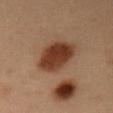<record>
<biopsy_status>not biopsied; imaged during a skin examination</biopsy_status>
<image>
  <source>total-body photography crop</source>
  <field_of_view_mm>15</field_of_view_mm>
</image>
<lighting>cross-polarized</lighting>
<automated_metrics>
  <eccentricity>0.7</eccentricity>
  <shape_asymmetry>0.15</shape_asymmetry>
</automated_metrics>
<site>mid back</site>
<lesion_size>
  <long_diameter_mm_approx>5.0</long_diameter_mm_approx>
</lesion_size>
<patient>
  <sex>male</sex>
  <age_approx>55</age_approx>
</patient>
</record>